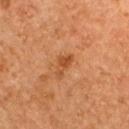The lesion was tiled from a total-body skin photograph and was not biopsied.
This image is a 15 mm lesion crop taken from a total-body photograph.
The patient is a female about 60 years old.
From the upper back.